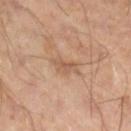follow-up — imaged on a skin check; not biopsied
image source — total-body-photography crop, ~15 mm field of view
body site — the leg
image-analysis metrics — a footprint of about 3 mm² and a shape eccentricity near 0.85; an average lesion color of about L≈55 a*≈19 b*≈31 (CIELAB), a lesion–skin lightness drop of about 8, and a lesion-to-skin contrast of about 5.5 (normalized; higher = more distinct)
lighting — cross-polarized
patient — male, approximately 45 years of age
lesion diameter — about 3 mm